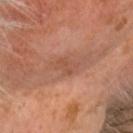Q: Is there a histopathology result?
A: no biopsy performed (imaged during a skin exam)
Q: How was the tile lit?
A: cross-polarized
Q: Lesion size?
A: ≈2.5 mm
Q: What are the patient's age and sex?
A: male, aged around 65
Q: What kind of image is this?
A: ~15 mm tile from a whole-body skin photo